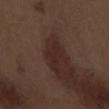Q: Is there a histopathology result?
A: no biopsy performed (imaged during a skin exam)
Q: Automated lesion metrics?
A: a footprint of about 3.5 mm² and an outline eccentricity of about 0.85 (0 = round, 1 = elongated); a classifier nevus-likeness of about 5/100 and a detector confidence of about 100 out of 100 that the crop contains a lesion
Q: Lesion location?
A: the left forearm
Q: How was this image acquired?
A: 15 mm crop, total-body photography
Q: What is the lesion's diameter?
A: about 3 mm
Q: What are the patient's age and sex?
A: male, aged around 70
Q: Illumination type?
A: white-light